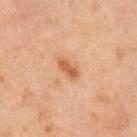{
  "biopsy_status": "not biopsied; imaged during a skin examination",
  "patient": {
    "sex": "male",
    "age_approx": 70
  },
  "lighting": "cross-polarized",
  "lesion_size": {
    "long_diameter_mm_approx": 3.0
  },
  "image": {
    "source": "total-body photography crop",
    "field_of_view_mm": 15
  },
  "site": "right upper arm"
}